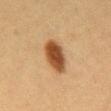Findings:
• workup — imaged on a skin check; not biopsied
• subject — female, aged 38–42
• illumination — cross-polarized illumination
• image source — ~15 mm tile from a whole-body skin photo
• automated metrics — a footprint of about 10 mm² and two-axis asymmetry of about 0.1; a lesion–skin lightness drop of about 15 and a normalized border contrast of about 11.5; a classifier nevus-likeness of about 100/100 and a detector confidence of about 100 out of 100 that the crop contains a lesion
• size — ~4.5 mm (longest diameter)
• body site — the back